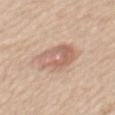Captured during whole-body skin photography for melanoma surveillance; the lesion was not biopsied.
An algorithmic analysis of the crop reported a color-variation rating of about 5.5/10 and peripheral color asymmetry of about 2. And it measured a nevus-likeness score of about 10/100.
From the back.
A roughly 15 mm field-of-view crop from a total-body skin photograph.
The tile uses white-light illumination.
A male subject aged 58 to 62.